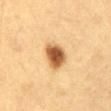<tbp_lesion>
<biopsy_status>not biopsied; imaged during a skin examination</biopsy_status>
<image>
  <source>total-body photography crop</source>
  <field_of_view_mm>15</field_of_view_mm>
</image>
<automated_metrics>
  <area_mm2_approx>9.0</area_mm2_approx>
  <border_irregularity_0_10>2.0</border_irregularity_0_10>
  <color_variation_0_10>5.5</color_variation_0_10>
  <peripheral_color_asymmetry>1.5</peripheral_color_asymmetry>
  <nevus_likeness_0_100>100</nevus_likeness_0_100>
</automated_metrics>
<patient>
  <sex>female</sex>
  <age_approx>30</age_approx>
</patient>
<site>abdomen</site>
<lesion_size>
  <long_diameter_mm_approx>4.0</long_diameter_mm_approx>
</lesion_size>
<lighting>cross-polarized</lighting>
</tbp_lesion>